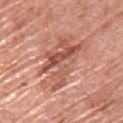Part of a total-body skin-imaging series; this lesion was reviewed on a skin check and was not flagged for biopsy.
The lesion's longest dimension is about 8 mm.
The subject is a female approximately 60 years of age.
Cropped from a total-body skin-imaging series; the visible field is about 15 mm.
Captured under white-light illumination.
From the upper back.